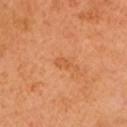Assessment:
Captured during whole-body skin photography for melanoma surveillance; the lesion was not biopsied.